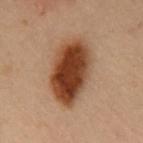Acquisition and patient details: The patient is a male aged around 55. A 15 mm crop from a total-body photograph taken for skin-cancer surveillance. Located on the chest.